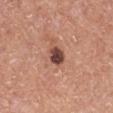follow-up: imaged on a skin check; not biopsied
lesion size: ≈2.5 mm
body site: the right lower leg
patient: male, aged around 55
image source: 15 mm crop, total-body photography
tile lighting: white-light illumination